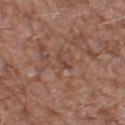Impression: The lesion was tiled from a total-body skin photograph and was not biopsied. Acquisition and patient details: A male subject aged around 60. This is a white-light tile. The lesion is located on the leg. A region of skin cropped from a whole-body photographic capture, roughly 15 mm wide. Longest diameter approximately 2.5 mm.